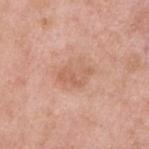Impression:
The lesion was tiled from a total-body skin photograph and was not biopsied.
Background:
Measured at roughly 4 mm in maximum diameter. Automated tile analysis of the lesion measured a border-irregularity index near 3.5/10, a within-lesion color-variation index near 3/10, and radial color variation of about 1. The analysis additionally found a nevus-likeness score of about 0/100 and lesion-presence confidence of about 100/100. On the back. A region of skin cropped from a whole-body photographic capture, roughly 15 mm wide. The subject is a male aged 53–57.